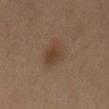{"biopsy_status": "not biopsied; imaged during a skin examination", "lighting": "cross-polarized", "site": "chest", "image": {"source": "total-body photography crop", "field_of_view_mm": 15}, "patient": {"sex": "male", "age_approx": 55}, "lesion_size": {"long_diameter_mm_approx": 4.5}, "automated_metrics": {"area_mm2_approx": 8.0, "eccentricity": 0.85, "shape_asymmetry": 0.25, "cielab_L": 41, "cielab_a": 16, "cielab_b": 28, "vs_skin_darker_L": 7.0, "border_irregularity_0_10": 2.5, "peripheral_color_asymmetry": 1.0, "nevus_likeness_0_100": 75, "lesion_detection_confidence_0_100": 100}}